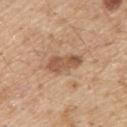Q: Was this lesion biopsied?
A: total-body-photography surveillance lesion; no biopsy
Q: Automated lesion metrics?
A: border irregularity of about 3 on a 0–10 scale, a within-lesion color-variation index near 3.5/10, and radial color variation of about 1; a classifier nevus-likeness of about 45/100 and lesion-presence confidence of about 100/100
Q: Lesion size?
A: about 4.5 mm
Q: What kind of image is this?
A: total-body-photography crop, ~15 mm field of view
Q: Illumination type?
A: white-light illumination
Q: Lesion location?
A: the upper back
Q: Patient demographics?
A: male, roughly 70 years of age